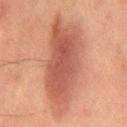follow-up — no biopsy performed (imaged during a skin exam) | automated lesion analysis — a lesion area of about 40 mm²; about 10 CIELAB-L* units darker than the surrounding skin and a lesion-to-skin contrast of about 8 (normalized; higher = more distinct); a border-irregularity index near 3/10, a color-variation rating of about 4/10, and peripheral color asymmetry of about 1.5 | tile lighting — cross-polarized illumination | anatomic site — the abdomen | patient — male, aged 63 to 67 | imaging modality — 15 mm crop, total-body photography.